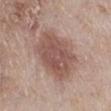{"biopsy_status": "not biopsied; imaged during a skin examination", "automated_metrics": {"eccentricity": 0.65, "shape_asymmetry": 0.15, "cielab_L": 53, "cielab_a": 19, "cielab_b": 23, "vs_skin_darker_L": 11.0, "vs_skin_contrast_norm": 8.0}, "lighting": "white-light", "image": {"source": "total-body photography crop", "field_of_view_mm": 15}, "lesion_size": {"long_diameter_mm_approx": 6.5}, "patient": {"sex": "male", "age_approx": 60}, "site": "right lower leg"}